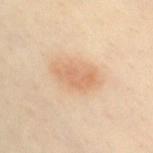  biopsy_status: not biopsied; imaged during a skin examination
  site: chest
  lighting: cross-polarized
  lesion_size:
    long_diameter_mm_approx: 5.0
  image:
    source: total-body photography crop
    field_of_view_mm: 15
  automated_metrics:
    eccentricity: 0.9
    cielab_L: 68
    cielab_a: 20
    cielab_b: 36
    vs_skin_darker_L: 9.0
    vs_skin_contrast_norm: 6.0
    nevus_likeness_0_100: 95
    lesion_detection_confidence_0_100: 100
  patient:
    sex: female
    age_approx: 40